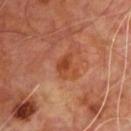– acquisition: total-body-photography crop, ~15 mm field of view
– anatomic site: the upper back
– TBP lesion metrics: a border-irregularity rating of about 3/10 and peripheral color asymmetry of about 0.5
– patient: male, aged 58 to 62
– lesion diameter: ~3 mm (longest diameter)